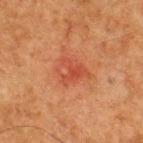Findings:
- biopsy status · no biopsy performed (imaged during a skin exam)
- patient · male, aged around 65
- size · ≈3.5 mm
- illumination · cross-polarized illumination
- automated lesion analysis · a lesion area of about 7.5 mm² and a shape eccentricity near 0.6; border irregularity of about 3 on a 0–10 scale, a color-variation rating of about 5/10, and a peripheral color-asymmetry measure near 1.5; a nevus-likeness score of about 0/100
- anatomic site · the upper back
- imaging modality · total-body-photography crop, ~15 mm field of view